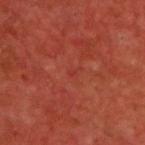Impression:
The lesion was photographed on a routine skin check and not biopsied; there is no pathology result.